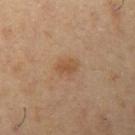biopsy status = catalogued during a skin exam; not biopsied
anatomic site = the left upper arm
automated metrics = an area of roughly 4 mm², an eccentricity of roughly 0.7, and two-axis asymmetry of about 0.2; a border-irregularity rating of about 2/10 and a within-lesion color-variation index near 2/10; a nevus-likeness score of about 45/100 and a detector confidence of about 100 out of 100 that the crop contains a lesion
illumination = cross-polarized illumination
acquisition = ~15 mm tile from a whole-body skin photo
subject = male, in their mid-50s